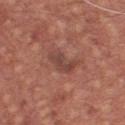Q: Was this lesion biopsied?
A: imaged on a skin check; not biopsied
Q: What lighting was used for the tile?
A: white-light
Q: Patient demographics?
A: male, roughly 65 years of age
Q: Lesion size?
A: ~4 mm (longest diameter)
Q: What is the anatomic site?
A: the front of the torso
Q: How was this image acquired?
A: 15 mm crop, total-body photography
Q: What did automated image analysis measure?
A: a lesion area of about 6.5 mm², a shape eccentricity near 0.85, and a symmetry-axis asymmetry near 0.3; an automated nevus-likeness rating near 0 out of 100